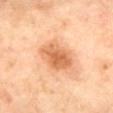Recorded during total-body skin imaging; not selected for excision or biopsy. The tile uses cross-polarized illumination. A female subject aged 58–62. About 5 mm across. The lesion is on the mid back. A 15 mm close-up tile from a total-body photography series done for melanoma screening.